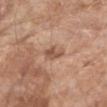Acquisition and patient details: The tile uses white-light illumination. A region of skin cropped from a whole-body photographic capture, roughly 15 mm wide. The patient is a female about 85 years old or older. Automated tile analysis of the lesion measured a lesion area of about 4.5 mm² and a symmetry-axis asymmetry near 0.3. The analysis additionally found a classifier nevus-likeness of about 0/100 and a detector confidence of about 100 out of 100 that the crop contains a lesion. Approximately 3 mm at its widest. Located on the right upper arm.